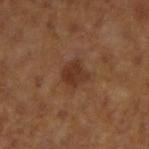Captured during whole-body skin photography for melanoma surveillance; the lesion was not biopsied.
Automated tile analysis of the lesion measured an eccentricity of roughly 0.15 and a symmetry-axis asymmetry near 0.25. The analysis additionally found a lesion–skin lightness drop of about 8 and a normalized border contrast of about 7.5. And it measured a nevus-likeness score of about 35/100.
A close-up tile cropped from a whole-body skin photograph, about 15 mm across.
The lesion is on the right forearm.
Longest diameter approximately 2.5 mm.
A male patient in their 60s.